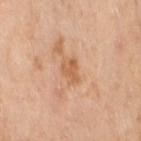The lesion was photographed on a routine skin check and not biopsied; there is no pathology result. A region of skin cropped from a whole-body photographic capture, roughly 15 mm wide. The recorded lesion diameter is about 3 mm. Captured under cross-polarized illumination. The lesion is located on the right thigh. A female patient, roughly 55 years of age.